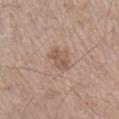biopsy status: total-body-photography surveillance lesion; no biopsy | acquisition: total-body-photography crop, ~15 mm field of view | patient: male, aged around 70 | diameter: ~3.5 mm (longest diameter) | site: the right lower leg.